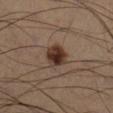Impression: No biopsy was performed on this lesion — it was imaged during a full skin examination and was not determined to be concerning. Acquisition and patient details: A region of skin cropped from a whole-body photographic capture, roughly 15 mm wide. The lesion is located on the leg. The tile uses cross-polarized illumination. A male subject, approximately 65 years of age. An algorithmic analysis of the crop reported an area of roughly 7.5 mm², a shape eccentricity near 0.4, and two-axis asymmetry of about 0.2. The software also gave a border-irregularity index near 2/10, a color-variation rating of about 5/10, and a peripheral color-asymmetry measure near 1.5.